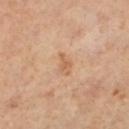follow-up: catalogued during a skin exam; not biopsied | diameter: ≈3 mm | subject: female, in their 60s | illumination: cross-polarized illumination | acquisition: ~15 mm crop, total-body skin-cancer survey | anatomic site: the right leg.